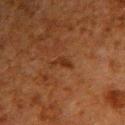  biopsy_status: not biopsied; imaged during a skin examination